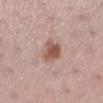Assessment:
Captured during whole-body skin photography for melanoma surveillance; the lesion was not biopsied.
Clinical summary:
Approximately 3.5 mm at its widest. The subject is a female in their mid- to late 30s. A lesion tile, about 15 mm wide, cut from a 3D total-body photograph. Imaged with white-light lighting. The lesion is located on the left lower leg.